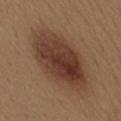Q: Is there a histopathology result?
A: no biopsy performed (imaged during a skin exam)
Q: Lesion location?
A: the mid back
Q: How was this image acquired?
A: ~15 mm crop, total-body skin-cancer survey
Q: What are the patient's age and sex?
A: female, aged approximately 40